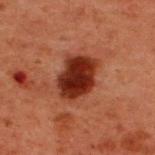{
  "image": {
    "source": "total-body photography crop",
    "field_of_view_mm": 15
  },
  "patient": {
    "sex": "male",
    "age_approx": 60
  },
  "site": "upper back"
}